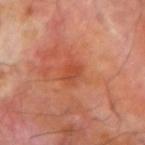Case summary:
* notes: no biopsy performed (imaged during a skin exam)
* image source: total-body-photography crop, ~15 mm field of view
* illumination: cross-polarized
* anatomic site: the left forearm
* image-analysis metrics: a classifier nevus-likeness of about 10/100
* lesion diameter: ~2.5 mm (longest diameter)
* subject: male, aged approximately 70